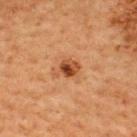<case>
<biopsy_status>not biopsied; imaged during a skin examination</biopsy_status>
</case>